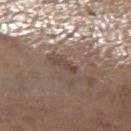Notes:
– workup · no biopsy performed (imaged during a skin exam)
– lesion size · ~2.5 mm (longest diameter)
– image source · total-body-photography crop, ~15 mm field of view
– body site · the left forearm
– patient · male, aged 68 to 72